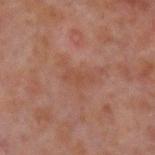workup: total-body-photography surveillance lesion; no biopsy | illumination: cross-polarized | site: the left forearm | acquisition: ~15 mm crop, total-body skin-cancer survey | automated metrics: a lesion area of about 3.5 mm² and a shape-asymmetry score of about 0.4 (0 = symmetric); a border-irregularity index near 4.5/10, internal color variation of about 0.5 on a 0–10 scale, and radial color variation of about 0 | lesion size: ≈3 mm | patient: male, aged 28–32.